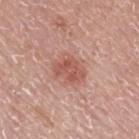Impression: Imaged during a routine full-body skin examination; the lesion was not biopsied and no histopathology is available. Image and clinical context: From the mid back. This is a white-light tile. A region of skin cropped from a whole-body photographic capture, roughly 15 mm wide. The lesion-visualizer software estimated a lesion area of about 8.5 mm², an outline eccentricity of about 0.65 (0 = round, 1 = elongated), and a shape-asymmetry score of about 0.25 (0 = symmetric). And it measured an average lesion color of about L≈55 a*≈26 b*≈27 (CIELAB), about 9 CIELAB-L* units darker than the surrounding skin, and a normalized border contrast of about 6.5. It also reported border irregularity of about 3 on a 0–10 scale. It also reported a detector confidence of about 100 out of 100 that the crop contains a lesion. The recorded lesion diameter is about 4 mm. A male subject, about 75 years old.